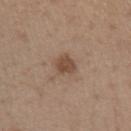{"lesion_size": {"long_diameter_mm_approx": 2.5}, "patient": {"sex": "female", "age_approx": 40}, "image": {"source": "total-body photography crop", "field_of_view_mm": 15}, "site": "left forearm"}